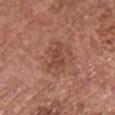Findings:
– workup · imaged on a skin check; not biopsied
– lesion size · ~4 mm (longest diameter)
– anatomic site · the chest
– subject · female, approximately 65 years of age
– image source · 15 mm crop, total-body photography
– illumination · white-light illumination